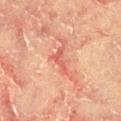follow-up: imaged on a skin check; not biopsied | body site: the right thigh | diameter: about 3.5 mm | automated lesion analysis: a footprint of about 4 mm², an eccentricity of roughly 0.9, and a shape-asymmetry score of about 0.5 (0 = symmetric); a mean CIELAB color near L≈55 a*≈31 b*≈30 and a normalized border contrast of about 6; a border-irregularity rating of about 6/10, a color-variation rating of about 0/10, and radial color variation of about 0; a classifier nevus-likeness of about 0/100 and lesion-presence confidence of about 65/100 | patient: male, in their mid- to late 60s | image source: ~15 mm crop, total-body skin-cancer survey.